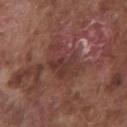Captured during whole-body skin photography for melanoma surveillance; the lesion was not biopsied. The lesion is located on the chest. Automated tile analysis of the lesion measured an area of roughly 12 mm², an outline eccentricity of about 0.8 (0 = round, 1 = elongated), and two-axis asymmetry of about 0.4. The software also gave a border-irregularity index near 5/10, internal color variation of about 3 on a 0–10 scale, and peripheral color asymmetry of about 1. The analysis additionally found a classifier nevus-likeness of about 0/100 and lesion-presence confidence of about 80/100. Cropped from a whole-body photographic skin survey; the tile spans about 15 mm. Imaged with white-light lighting. The subject is a male aged 73–77.